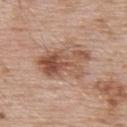The lesion was photographed on a routine skin check and not biopsied; there is no pathology result.
This image is a 15 mm lesion crop taken from a total-body photograph.
About 6.5 mm across.
A male subject approximately 55 years of age.
From the upper back.